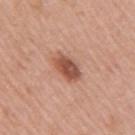This lesion was catalogued during total-body skin photography and was not selected for biopsy.
Captured under white-light illumination.
A female patient, aged around 40.
The lesion's longest dimension is about 4 mm.
An algorithmic analysis of the crop reported a lesion area of about 8 mm², an outline eccentricity of about 0.75 (0 = round, 1 = elongated), and a symmetry-axis asymmetry near 0.15. The software also gave a lesion color around L≈53 a*≈24 b*≈30 in CIELAB, a lesion–skin lightness drop of about 13, and a lesion-to-skin contrast of about 9 (normalized; higher = more distinct).
A 15 mm close-up tile from a total-body photography series done for melanoma screening.
On the right upper arm.